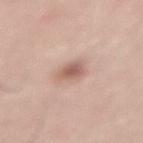* biopsy status: catalogued during a skin exam; not biopsied
* TBP lesion metrics: a border-irregularity index near 2/10, a color-variation rating of about 2.5/10, and peripheral color asymmetry of about 0.5
* body site: the lower back
* patient: male, aged around 60
* lighting: white-light illumination
* imaging modality: 15 mm crop, total-body photography
* size: ~2.5 mm (longest diameter)